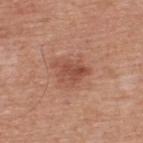workup=catalogued during a skin exam; not biopsied
subject=male, in their mid- to late 50s
anatomic site=the upper back
imaging modality=~15 mm tile from a whole-body skin photo
illumination=white-light
diameter=~4 mm (longest diameter)